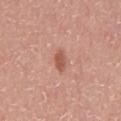Background: The tile uses white-light illumination. Cropped from a total-body skin-imaging series; the visible field is about 15 mm. Longest diameter approximately 3 mm. A male patient aged around 45. An algorithmic analysis of the crop reported an area of roughly 3.5 mm², an eccentricity of roughly 0.85, and a symmetry-axis asymmetry near 0.25. Located on the front of the torso.